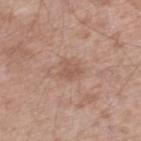No biopsy was performed on this lesion — it was imaged during a full skin examination and was not determined to be concerning.
A male patient, about 45 years old.
The lesion is located on the right lower leg.
A region of skin cropped from a whole-body photographic capture, roughly 15 mm wide.